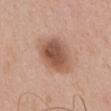Impression: Recorded during total-body skin imaging; not selected for excision or biopsy. Background: The total-body-photography lesion software estimated a lesion area of about 16 mm², an outline eccentricity of about 0.5 (0 = round, 1 = elongated), and two-axis asymmetry of about 0.2. The software also gave a lesion color around L≈54 a*≈21 b*≈29 in CIELAB, roughly 13 lightness units darker than nearby skin, and a lesion-to-skin contrast of about 9 (normalized; higher = more distinct). The software also gave border irregularity of about 1.5 on a 0–10 scale and radial color variation of about 1.5. From the chest. This image is a 15 mm lesion crop taken from a total-body photograph. The subject is a male roughly 60 years of age.